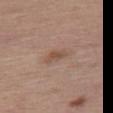Assessment:
Part of a total-body skin-imaging series; this lesion was reviewed on a skin check and was not flagged for biopsy.
Image and clinical context:
A female patient roughly 65 years of age. Cropped from a whole-body photographic skin survey; the tile spans about 15 mm. The total-body-photography lesion software estimated a border-irregularity index near 4/10. It also reported a lesion-detection confidence of about 100/100. Located on the left leg.